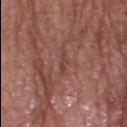No biopsy was performed on this lesion — it was imaged during a full skin examination and was not determined to be concerning. A roughly 15 mm field-of-view crop from a total-body skin photograph. A male patient, aged 63–67. Located on the head or neck. Captured under white-light illumination. Measured at roughly 3 mm in maximum diameter.